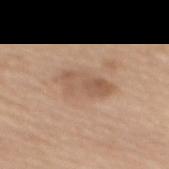Notes:
– subject · female, aged 68–72
– size · about 5.5 mm
– location · the mid back
– automated lesion analysis · border irregularity of about 3 on a 0–10 scale, a within-lesion color-variation index near 4/10, and peripheral color asymmetry of about 1.5
– image · ~15 mm tile from a whole-body skin photo